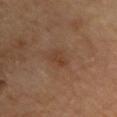Recorded during total-body skin imaging; not selected for excision or biopsy. Longest diameter approximately 3 mm. The lesion is located on the chest. A region of skin cropped from a whole-body photographic capture, roughly 15 mm wide. The tile uses cross-polarized illumination.